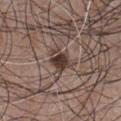follow-up: catalogued during a skin exam; not biopsied
subject: male, roughly 50 years of age
automated metrics: a footprint of about 6 mm², an eccentricity of roughly 0.6, and two-axis asymmetry of about 0.3; about 13 CIELAB-L* units darker than the surrounding skin and a lesion-to-skin contrast of about 10.5 (normalized; higher = more distinct); a border-irregularity rating of about 3/10, a within-lesion color-variation index near 4.5/10, and radial color variation of about 1.5; a classifier nevus-likeness of about 95/100
tile lighting: white-light illumination
anatomic site: the chest
acquisition: ~15 mm crop, total-body skin-cancer survey